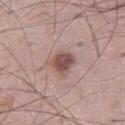The lesion was photographed on a routine skin check and not biopsied; there is no pathology result. A male subject, about 50 years old. Measured at roughly 3 mm in maximum diameter. An algorithmic analysis of the crop reported a classifier nevus-likeness of about 75/100 and lesion-presence confidence of about 100/100. On the leg. Cropped from a whole-body photographic skin survey; the tile spans about 15 mm.